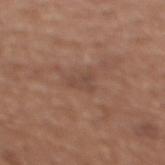| field | value |
|---|---|
| biopsy status | total-body-photography surveillance lesion; no biopsy |
| subject | female, in their mid- to late 30s |
| illumination | white-light |
| acquisition | ~15 mm tile from a whole-body skin photo |
| lesion diameter | about 2.5 mm |
| image-analysis metrics | a footprint of about 3.5 mm², an eccentricity of roughly 0.8, and two-axis asymmetry of about 0.4; an average lesion color of about L≈46 a*≈19 b*≈26 (CIELAB), a lesion–skin lightness drop of about 6, and a normalized border contrast of about 5; a border-irregularity index near 4/10 and a within-lesion color-variation index near 1/10; a classifier nevus-likeness of about 0/100 and a lesion-detection confidence of about 100/100 |
| site | the chest |